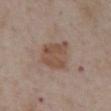Captured during whole-body skin photography for melanoma surveillance; the lesion was not biopsied.